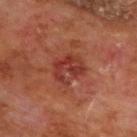Impression:
The lesion was tiled from a total-body skin photograph and was not biopsied.